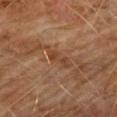– workup — total-body-photography surveillance lesion; no biopsy